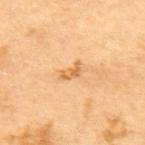Image and clinical context:
A 15 mm crop from a total-body photograph taken for skin-cancer surveillance. The lesion is on the upper back. The patient is a female in their mid- to late 60s. The tile uses cross-polarized illumination. The lesion's longest dimension is about 2.5 mm.